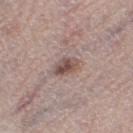<tbp_lesion>
<biopsy_status>not biopsied; imaged during a skin examination</biopsy_status>
<image>
  <source>total-body photography crop</source>
  <field_of_view_mm>15</field_of_view_mm>
</image>
<patient>
  <sex>female</sex>
  <age_approx>65</age_approx>
</patient>
<site>left thigh</site>
</tbp_lesion>